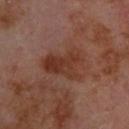Impression:
This lesion was catalogued during total-body skin photography and was not selected for biopsy.
Image and clinical context:
Captured under cross-polarized illumination. A close-up tile cropped from a whole-body skin photograph, about 15 mm across. A male patient, in their 70s. From the back.